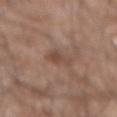Findings:
• biopsy status: no biopsy performed (imaged during a skin exam)
• anatomic site: the mid back
• tile lighting: white-light
• size: ≈3 mm
• subject: male, roughly 60 years of age
• acquisition: total-body-photography crop, ~15 mm field of view